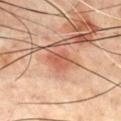Clinical impression: The lesion was photographed on a routine skin check and not biopsied; there is no pathology result. Acquisition and patient details: The recorded lesion diameter is about 3.5 mm. A roughly 15 mm field-of-view crop from a total-body skin photograph. The patient is a male aged 68–72. The lesion is on the chest. The tile uses cross-polarized illumination.